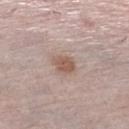biopsy_status: not biopsied; imaged during a skin examination
site: left lower leg
lesion_size:
  long_diameter_mm_approx: 3.0
patient:
  sex: female
  age_approx: 65
image:
  source: total-body photography crop
  field_of_view_mm: 15